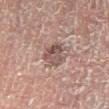Context: A 15 mm close-up extracted from a 3D total-body photography capture. From the left lower leg. The patient is a male roughly 50 years of age. An algorithmic analysis of the crop reported an average lesion color of about L≈45 a*≈16 b*≈19 (CIELAB). The software also gave a border-irregularity rating of about 2.5/10, a color-variation rating of about 5.5/10, and a peripheral color-asymmetry measure near 2. The analysis additionally found an automated nevus-likeness rating near 0 out of 100 and a lesion-detection confidence of about 100/100.